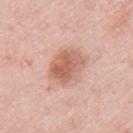Case summary:
- follow-up: total-body-photography surveillance lesion; no biopsy
- patient: female, in their mid- to late 60s
- diameter: about 4.5 mm
- TBP lesion metrics: border irregularity of about 2 on a 0–10 scale and radial color variation of about 1.5; an automated nevus-likeness rating near 70 out of 100 and a lesion-detection confidence of about 100/100
- acquisition: 15 mm crop, total-body photography
- site: the left upper arm